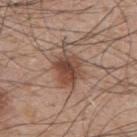| key | value |
|---|---|
| follow-up | no biopsy performed (imaged during a skin exam) |
| location | the upper back |
| lesion size | ≈5.5 mm |
| patient | male, aged 48 to 52 |
| tile lighting | white-light |
| imaging modality | ~15 mm tile from a whole-body skin photo |